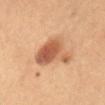subject = female, aged 58 to 62
lesion diameter = about 5.5 mm
site = the abdomen
imaging modality = ~15 mm tile from a whole-body skin photo